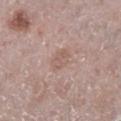Impression:
The lesion was photographed on a routine skin check and not biopsied; there is no pathology result.
Context:
Cropped from a total-body skin-imaging series; the visible field is about 15 mm. This is a white-light tile. The lesion is on the left lower leg. A female subject, approximately 65 years of age. Approximately 3 mm at its widest.